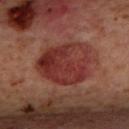Recorded during total-body skin imaging; not selected for excision or biopsy. The recorded lesion diameter is about 6.5 mm. This is a cross-polarized tile. The lesion is located on the upper back. A roughly 15 mm field-of-view crop from a total-body skin photograph. A female subject, approximately 45 years of age.